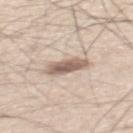Part of a total-body skin-imaging series; this lesion was reviewed on a skin check and was not flagged for biopsy. This is a white-light tile. Cropped from a total-body skin-imaging series; the visible field is about 15 mm. The subject is a male aged 58–62. The lesion's longest dimension is about 4.5 mm. Located on the mid back.